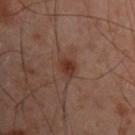  lesion_size:
    long_diameter_mm_approx: 2.5
  lighting: cross-polarized
  site: left arm
  patient:
    sex: male
    age_approx: 50
  image:
    source: total-body photography crop
    field_of_view_mm: 15